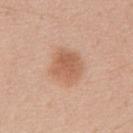Assessment:
Imaged during a routine full-body skin examination; the lesion was not biopsied and no histopathology is available.
Acquisition and patient details:
Cropped from a whole-body photographic skin survey; the tile spans about 15 mm. A male subject, approximately 50 years of age. Imaged with white-light lighting. Measured at roughly 4 mm in maximum diameter. On the front of the torso.